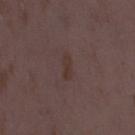Imaged during a routine full-body skin examination; the lesion was not biopsied and no histopathology is available.